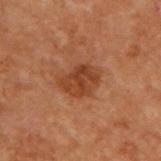Assessment:
The lesion was tiled from a total-body skin photograph and was not biopsied.
Acquisition and patient details:
The lesion is located on the upper back. A lesion tile, about 15 mm wide, cut from a 3D total-body photograph. The patient is a male about 70 years old. The recorded lesion diameter is about 4 mm. The lesion-visualizer software estimated an area of roughly 9.5 mm² and two-axis asymmetry of about 0.2. And it measured a lesion color around L≈31 a*≈20 b*≈28 in CIELAB, about 8 CIELAB-L* units darker than the surrounding skin, and a normalized border contrast of about 7.5. It also reported a classifier nevus-likeness of about 65/100 and a detector confidence of about 100 out of 100 that the crop contains a lesion.